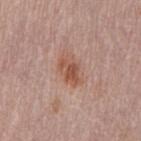Impression:
The lesion was photographed on a routine skin check and not biopsied; there is no pathology result.
Context:
Longest diameter approximately 4 mm. The tile uses white-light illumination. A female patient in their 50s. The lesion is on the left thigh. A 15 mm crop from a total-body photograph taken for skin-cancer surveillance. The total-body-photography lesion software estimated a footprint of about 7.5 mm² and an outline eccentricity of about 0.8 (0 = round, 1 = elongated). And it measured border irregularity of about 2 on a 0–10 scale, a color-variation rating of about 5/10, and a peripheral color-asymmetry measure near 1.5.